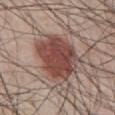Part of a total-body skin-imaging series; this lesion was reviewed on a skin check and was not flagged for biopsy. An algorithmic analysis of the crop reported a lesion color around L≈45 a*≈21 b*≈23 in CIELAB. It also reported radial color variation of about 1.5. Cropped from a total-body skin-imaging series; the visible field is about 15 mm. Approximately 6 mm at its widest. Located on the abdomen. A male subject, aged 48 to 52.